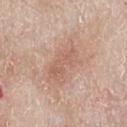Image and clinical context:
A male patient aged 63–67. The lesion is located on the chest. This is a white-light tile. This image is a 15 mm lesion crop taken from a total-body photograph. Longest diameter approximately 5.5 mm.